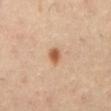Impression: Part of a total-body skin-imaging series; this lesion was reviewed on a skin check and was not flagged for biopsy. Acquisition and patient details: Imaged with cross-polarized lighting. A 15 mm crop from a total-body photograph taken for skin-cancer surveillance. Approximately 2.5 mm at its widest. A male patient in their mid-50s. From the mid back. Automated tile analysis of the lesion measured a border-irregularity rating of about 2/10, internal color variation of about 2.5 on a 0–10 scale, and peripheral color asymmetry of about 1. The analysis additionally found an automated nevus-likeness rating near 95 out of 100 and lesion-presence confidence of about 100/100.